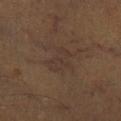– follow-up — imaged on a skin check; not biopsied
– anatomic site — the left lower leg
– subject — male, in their 60s
– image source — ~15 mm tile from a whole-body skin photo